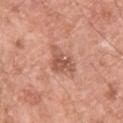Clinical summary:
A 15 mm crop from a total-body photograph taken for skin-cancer surveillance. The tile uses white-light illumination. The patient is a male roughly 55 years of age. The lesion is located on the upper back. Approximately 3.5 mm at its widest.